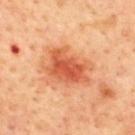Q: Was this lesion biopsied?
A: total-body-photography surveillance lesion; no biopsy
Q: Lesion size?
A: ≈6 mm
Q: Who is the patient?
A: male, aged approximately 65
Q: What is the imaging modality?
A: ~15 mm crop, total-body skin-cancer survey
Q: Lesion location?
A: the upper back
Q: How was the tile lit?
A: cross-polarized illumination